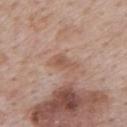{
  "biopsy_status": "not biopsied; imaged during a skin examination",
  "image": {
    "source": "total-body photography crop",
    "field_of_view_mm": 15
  },
  "patient": {
    "sex": "male",
    "age_approx": 75
  },
  "lesion_size": {
    "long_diameter_mm_approx": 3.5
  },
  "site": "mid back",
  "lighting": "white-light"
}